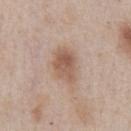Assessment:
No biopsy was performed on this lesion — it was imaged during a full skin examination and was not determined to be concerning.
Acquisition and patient details:
About 4 mm across. Captured under white-light illumination. A 15 mm crop from a total-body photograph taken for skin-cancer surveillance. Located on the chest. The patient is a male aged approximately 60.